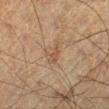notes: catalogued during a skin exam; not biopsied
lighting: cross-polarized illumination
image-analysis metrics: a border-irregularity index near 3.5/10 and internal color variation of about 1.5 on a 0–10 scale
lesion size: ~3 mm (longest diameter)
anatomic site: the leg
subject: male, aged approximately 60
imaging modality: ~15 mm tile from a whole-body skin photo